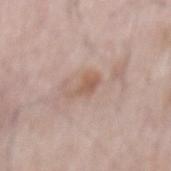Imaged during a routine full-body skin examination; the lesion was not biopsied and no histopathology is available. On the mid back. A close-up tile cropped from a whole-body skin photograph, about 15 mm across. A male patient about 65 years old.